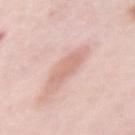Clinical impression: Captured during whole-body skin photography for melanoma surveillance; the lesion was not biopsied. Acquisition and patient details: A female subject, in their mid-60s. This image is a 15 mm lesion crop taken from a total-body photograph. The lesion is located on the left upper arm. Measured at roughly 3.5 mm in maximum diameter. Captured under white-light illumination. The lesion-visualizer software estimated a shape-asymmetry score of about 0.25 (0 = symmetric). It also reported border irregularity of about 3 on a 0–10 scale and a within-lesion color-variation index near 1.5/10. The software also gave a classifier nevus-likeness of about 45/100 and a detector confidence of about 100 out of 100 that the crop contains a lesion.